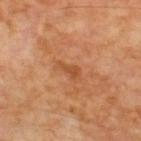patient: male, about 70 years old
image source: ~15 mm crop, total-body skin-cancer survey
body site: the upper back
TBP lesion metrics: a mean CIELAB color near L≈49 a*≈26 b*≈39, a lesion–skin lightness drop of about 8, and a normalized border contrast of about 6; internal color variation of about 0 on a 0–10 scale and radial color variation of about 0; an automated nevus-likeness rating near 0 out of 100 and lesion-presence confidence of about 100/100